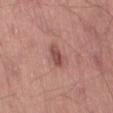No biopsy was performed on this lesion — it was imaged during a full skin examination and was not determined to be concerning.
The patient is a male approximately 55 years of age.
Captured under white-light illumination.
Automated image analysis of the tile measured a border-irregularity rating of about 2.5/10.
The recorded lesion diameter is about 3 mm.
A close-up tile cropped from a whole-body skin photograph, about 15 mm across.
The lesion is on the left thigh.